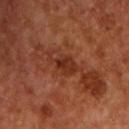{
  "biopsy_status": "not biopsied; imaged during a skin examination",
  "patient": {
    "sex": "male",
    "age_approx": 55
  },
  "automated_metrics": {
    "cielab_L": 29,
    "cielab_a": 24,
    "cielab_b": 29,
    "vs_skin_darker_L": 8.0,
    "vs_skin_contrast_norm": 7.5,
    "border_irregularity_0_10": 4.5,
    "color_variation_0_10": 3.0,
    "peripheral_color_asymmetry": 1.0
  },
  "lighting": "cross-polarized",
  "lesion_size": {
    "long_diameter_mm_approx": 4.0
  },
  "image": {
    "source": "total-body photography crop",
    "field_of_view_mm": 15
  },
  "site": "chest"
}